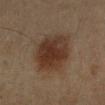Imaged during a routine full-body skin examination; the lesion was not biopsied and no histopathology is available. On the arm. The tile uses cross-polarized illumination. A 15 mm close-up tile from a total-body photography series done for melanoma screening. A female subject, about 45 years old. Automated tile analysis of the lesion measured border irregularity of about 1.5 on a 0–10 scale, a within-lesion color-variation index near 3.5/10, and radial color variation of about 1. Longest diameter approximately 5.5 mm.